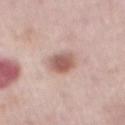<case>
  <biopsy_status>not biopsied; imaged during a skin examination</biopsy_status>
  <image>
    <source>total-body photography crop</source>
    <field_of_view_mm>15</field_of_view_mm>
  </image>
  <lesion_size>
    <long_diameter_mm_approx>3.5</long_diameter_mm_approx>
  </lesion_size>
  <patient>
    <sex>male</sex>
    <age_approx>75</age_approx>
  </patient>
  <site>abdomen</site>
  <automated_metrics>
    <border_irregularity_0_10>2.0</border_irregularity_0_10>
    <color_variation_0_10>4.5</color_variation_0_10>
    <peripheral_color_asymmetry>1.5</peripheral_color_asymmetry>
  </automated_metrics>
</case>